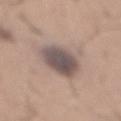Context:
Cropped from a whole-body photographic skin survey; the tile spans about 15 mm. The lesion is located on the mid back. A male patient, aged around 65.